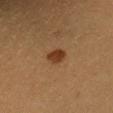Imaged during a routine full-body skin examination; the lesion was not biopsied and no histopathology is available. The subject is a female aged approximately 30. From the left forearm. Approximately 2.5 mm at its widest. A roughly 15 mm field-of-view crop from a total-body skin photograph.